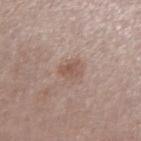Part of a total-body skin-imaging series; this lesion was reviewed on a skin check and was not flagged for biopsy.
The lesion is on the left forearm.
Captured under white-light illumination.
The patient is a female aged approximately 40.
The lesion-visualizer software estimated a nevus-likeness score of about 40/100 and a detector confidence of about 100 out of 100 that the crop contains a lesion.
A roughly 15 mm field-of-view crop from a total-body skin photograph.